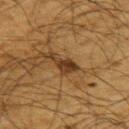<case>
<biopsy_status>not biopsied; imaged during a skin examination</biopsy_status>
<site>back</site>
<lesion_size>
  <long_diameter_mm_approx>4.0</long_diameter_mm_approx>
</lesion_size>
<patient>
  <sex>male</sex>
  <age_approx>65</age_approx>
</patient>
<automated_metrics>
  <cielab_L>29</cielab_L>
  <cielab_a>16</cielab_a>
  <cielab_b>28</cielab_b>
  <vs_skin_darker_L>10.0</vs_skin_darker_L>
  <vs_skin_contrast_norm>10.5</vs_skin_contrast_norm>
  <border_irregularity_0_10>4.5</border_irregularity_0_10>
  <color_variation_0_10>3.0</color_variation_0_10>
  <peripheral_color_asymmetry>1.0</peripheral_color_asymmetry>
  <lesion_detection_confidence_0_100>75</lesion_detection_confidence_0_100>
</automated_metrics>
<image>
  <source>total-body photography crop</source>
  <field_of_view_mm>15</field_of_view_mm>
</image>
</case>